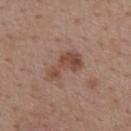Impression:
This lesion was catalogued during total-body skin photography and was not selected for biopsy.
Image and clinical context:
A female patient approximately 45 years of age. The lesion is located on the mid back. A close-up tile cropped from a whole-body skin photograph, about 15 mm across. Captured under white-light illumination. The recorded lesion diameter is about 4.5 mm.